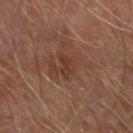No biopsy was performed on this lesion — it was imaged during a full skin examination and was not determined to be concerning.
The recorded lesion diameter is about 3 mm.
The lesion is located on the left lower leg.
A roughly 15 mm field-of-view crop from a total-body skin photograph.
The lesion-visualizer software estimated a lesion area of about 3.5 mm², an eccentricity of roughly 0.85, and a symmetry-axis asymmetry near 0.4. The software also gave an average lesion color of about L≈35 a*≈22 b*≈27 (CIELAB), about 6 CIELAB-L* units darker than the surrounding skin, and a normalized border contrast of about 6. The software also gave border irregularity of about 4.5 on a 0–10 scale, a within-lesion color-variation index near 1.5/10, and peripheral color asymmetry of about 0.5. It also reported a classifier nevus-likeness of about 0/100 and a detector confidence of about 95 out of 100 that the crop contains a lesion.
A male subject, aged 63–67.
Captured under cross-polarized illumination.